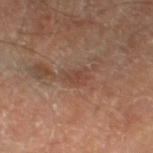Notes:
* notes — no biopsy performed (imaged during a skin exam)
* TBP lesion metrics — an area of roughly 3.5 mm² and a symmetry-axis asymmetry near 0.45; a mean CIELAB color near L≈42 a*≈19 b*≈26 and a lesion–skin lightness drop of about 7; border irregularity of about 5 on a 0–10 scale and a peripheral color-asymmetry measure near 0.5
* illumination — cross-polarized illumination
* size — ~2.5 mm (longest diameter)
* location — the left thigh
* acquisition — 15 mm crop, total-body photography
* subject — male, aged around 70